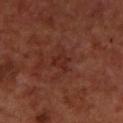Findings:
- anatomic site — the upper back
- subject — male, about 70 years old
- lesion size — ≈3 mm
- image-analysis metrics — a shape-asymmetry score of about 0.4 (0 = symmetric); roughly 5 lightness units darker than nearby skin and a lesion-to-skin contrast of about 5.5 (normalized; higher = more distinct); a nevus-likeness score of about 0/100
- image — ~15 mm tile from a whole-body skin photo